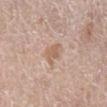biopsy status — imaged on a skin check; not biopsied
patient — male, about 60 years old
body site — the right lower leg
imaging modality — ~15 mm tile from a whole-body skin photo
lesion diameter — about 2.5 mm
TBP lesion metrics — a footprint of about 4.5 mm², an outline eccentricity of about 0.6 (0 = round, 1 = elongated), and two-axis asymmetry of about 0.35; a mean CIELAB color near L≈60 a*≈18 b*≈30, about 8 CIELAB-L* units darker than the surrounding skin, and a normalized border contrast of about 6.5